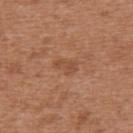• notes — no biopsy performed (imaged during a skin exam)
• anatomic site — the back
• automated metrics — a shape eccentricity near 0.85 and two-axis asymmetry of about 0.45; a lesion color around L≈49 a*≈23 b*≈33 in CIELAB, about 7 CIELAB-L* units darker than the surrounding skin, and a lesion-to-skin contrast of about 5.5 (normalized; higher = more distinct); a border-irregularity rating of about 4.5/10, internal color variation of about 0 on a 0–10 scale, and a peripheral color-asymmetry measure near 0; a nevus-likeness score of about 0/100 and a detector confidence of about 100 out of 100 that the crop contains a lesion
• acquisition — 15 mm crop, total-body photography
• diameter — about 2.5 mm
• subject — female, aged approximately 40